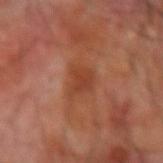Q: Was this lesion biopsied?
A: total-body-photography surveillance lesion; no biopsy
Q: How was this image acquired?
A: ~15 mm crop, total-body skin-cancer survey
Q: What did automated image analysis measure?
A: an area of roughly 6.5 mm² and two-axis asymmetry of about 0.4; a lesion color around L≈41 a*≈26 b*≈33 in CIELAB and a lesion–skin lightness drop of about 7
Q: How was the tile lit?
A: cross-polarized
Q: Patient demographics?
A: male, aged 68–72
Q: What is the anatomic site?
A: the right forearm